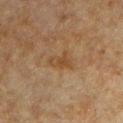workup = total-body-photography surveillance lesion; no biopsy
lesion diameter = about 3 mm
location = the chest
automated lesion analysis = border irregularity of about 5 on a 0–10 scale, internal color variation of about 2 on a 0–10 scale, and a peripheral color-asymmetry measure near 0.5; a lesion-detection confidence of about 100/100
image = ~15 mm tile from a whole-body skin photo
patient = male, aged 48 to 52
illumination = cross-polarized illumination